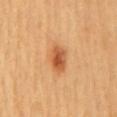This lesion was catalogued during total-body skin photography and was not selected for biopsy.
The tile uses cross-polarized illumination.
On the mid back.
A female patient in their 60s.
A roughly 15 mm field-of-view crop from a total-body skin photograph.
About 4 mm across.